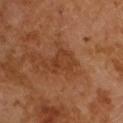No biopsy was performed on this lesion — it was imaged during a full skin examination and was not determined to be concerning. Measured at roughly 3 mm in maximum diameter. Captured under cross-polarized illumination. A male patient, about 65 years old. An algorithmic analysis of the crop reported a lesion area of about 6.5 mm², an outline eccentricity of about 0.65 (0 = round, 1 = elongated), and a symmetry-axis asymmetry near 0.4. The analysis additionally found border irregularity of about 4 on a 0–10 scale, internal color variation of about 3 on a 0–10 scale, and a peripheral color-asymmetry measure near 1. The analysis additionally found lesion-presence confidence of about 100/100. A 15 mm close-up tile from a total-body photography series done for melanoma screening.